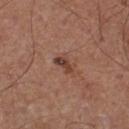Imaged during a routine full-body skin examination; the lesion was not biopsied and no histopathology is available. Approximately 2.5 mm at its widest. A male subject approximately 55 years of age. Cropped from a total-body skin-imaging series; the visible field is about 15 mm. Located on the chest.